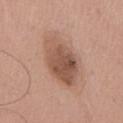The lesion was tiled from a total-body skin photograph and was not biopsied.
The patient is a male approximately 55 years of age.
The lesion is located on the chest.
Cropped from a total-body skin-imaging series; the visible field is about 15 mm.